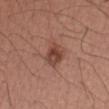lighting: white-light
image:
  source: total-body photography crop
  field_of_view_mm: 15
patient:
  sex: male
  age_approx: 55
site: left forearm
automated_metrics:
  cielab_L: 44
  cielab_a: 23
  cielab_b: 27
  vs_skin_darker_L: 10.0
  vs_skin_contrast_norm: 8.0
lesion_size:
  long_diameter_mm_approx: 3.5